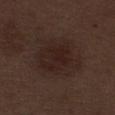Impression: Imaged during a routine full-body skin examination; the lesion was not biopsied and no histopathology is available. Clinical summary: A 15 mm close-up tile from a total-body photography series done for melanoma screening. The lesion is on the leg. The lesion-visualizer software estimated a footprint of about 29 mm², an outline eccentricity of about 0.3 (0 = round, 1 = elongated), and a symmetry-axis asymmetry near 0.15. It also reported a border-irregularity rating of about 2/10, internal color variation of about 3.5 on a 0–10 scale, and a peripheral color-asymmetry measure near 1. The software also gave a classifier nevus-likeness of about 50/100 and a detector confidence of about 100 out of 100 that the crop contains a lesion. Measured at roughly 6 mm in maximum diameter. The tile uses white-light illumination. The subject is a male aged around 70.